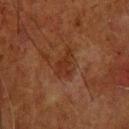A male subject aged 63 to 67.
From the head or neck.
Captured under cross-polarized illumination.
The total-body-photography lesion software estimated a lesion area of about 5.5 mm², an outline eccentricity of about 0.85 (0 = round, 1 = elongated), and a symmetry-axis asymmetry near 0.45. And it measured a lesion-detection confidence of about 100/100.
This image is a 15 mm lesion crop taken from a total-body photograph.
Longest diameter approximately 3.5 mm.